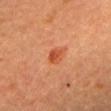Q: Was this lesion biopsied?
A: total-body-photography surveillance lesion; no biopsy
Q: Lesion size?
A: ≈2.5 mm
Q: What is the anatomic site?
A: the head or neck
Q: Patient demographics?
A: female, roughly 60 years of age
Q: What lighting was used for the tile?
A: cross-polarized
Q: How was this image acquired?
A: ~15 mm tile from a whole-body skin photo